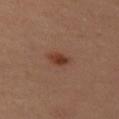The lesion was tiled from a total-body skin photograph and was not biopsied. From the leg. A 15 mm close-up extracted from a 3D total-body photography capture. The tile uses cross-polarized illumination. The subject is a female roughly 30 years of age. Longest diameter approximately 2.5 mm.